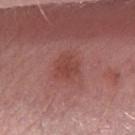Clinical impression: Part of a total-body skin-imaging series; this lesion was reviewed on a skin check and was not flagged for biopsy. Background: On the arm. Cropped from a total-body skin-imaging series; the visible field is about 15 mm. The recorded lesion diameter is about 3 mm. The patient is a female aged 43–47. The total-body-photography lesion software estimated about 7 CIELAB-L* units darker than the surrounding skin and a normalized lesion–skin contrast near 5.5. The software also gave border irregularity of about 3 on a 0–10 scale, a color-variation rating of about 2.5/10, and radial color variation of about 1. The analysis additionally found a classifier nevus-likeness of about 0/100 and lesion-presence confidence of about 100/100.